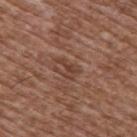Q: Was a biopsy performed?
A: catalogued during a skin exam; not biopsied
Q: What lighting was used for the tile?
A: white-light
Q: Patient demographics?
A: male, aged approximately 75
Q: What is the anatomic site?
A: the upper back
Q: How large is the lesion?
A: ≈3 mm
Q: Automated lesion metrics?
A: an area of roughly 3.5 mm² and an outline eccentricity of about 0.85 (0 = round, 1 = elongated); an automated nevus-likeness rating near 15 out of 100 and a lesion-detection confidence of about 85/100
Q: What is the imaging modality?
A: ~15 mm tile from a whole-body skin photo